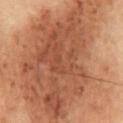Imaged during a routine full-body skin examination; the lesion was not biopsied and no histopathology is available. A lesion tile, about 15 mm wide, cut from a 3D total-body photograph. A male patient, aged approximately 55. Automated image analysis of the tile measured a mean CIELAB color near L≈44 a*≈20 b*≈28 and about 13 CIELAB-L* units darker than the surrounding skin. On the front of the torso. This is a cross-polarized tile.